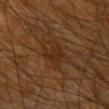Recorded during total-body skin imaging; not selected for excision or biopsy.
The tile uses cross-polarized illumination.
A close-up tile cropped from a whole-body skin photograph, about 15 mm across.
Located on the right upper arm.
The subject is a male aged 63 to 67.
The lesion-visualizer software estimated a footprint of about 6.5 mm², an outline eccentricity of about 0.85 (0 = round, 1 = elongated), and a symmetry-axis asymmetry near 0.4. The analysis additionally found a mean CIELAB color near L≈21 a*≈14 b*≈24, about 5 CIELAB-L* units darker than the surrounding skin, and a normalized lesion–skin contrast near 6. The software also gave a nevus-likeness score of about 0/100 and a detector confidence of about 75 out of 100 that the crop contains a lesion.